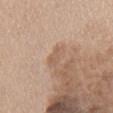Assessment:
Captured during whole-body skin photography for melanoma surveillance; the lesion was not biopsied.
Acquisition and patient details:
Cropped from a total-body skin-imaging series; the visible field is about 15 mm. The lesion is on the front of the torso. The recorded lesion diameter is about 3 mm. The total-body-photography lesion software estimated border irregularity of about 3 on a 0–10 scale, a color-variation rating of about 1.5/10, and a peripheral color-asymmetry measure near 0.5. A male subject aged approximately 60. Captured under white-light illumination.